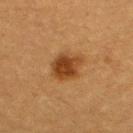• notes · no biopsy performed (imaged during a skin exam)
• acquisition · ~15 mm tile from a whole-body skin photo
• subject · female, aged approximately 55
• diameter · ~4.5 mm (longest diameter)
• lighting · cross-polarized
• anatomic site · the chest